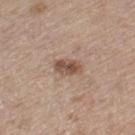The lesion was tiled from a total-body skin photograph and was not biopsied. Automated tile analysis of the lesion measured a lesion–skin lightness drop of about 12. And it measured a border-irregularity rating of about 2/10 and radial color variation of about 1.5. A lesion tile, about 15 mm wide, cut from a 3D total-body photograph. A male patient, roughly 70 years of age. The lesion is located on the left thigh. The recorded lesion diameter is about 3.5 mm.